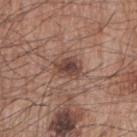No biopsy was performed on this lesion — it was imaged during a full skin examination and was not determined to be concerning.
An algorithmic analysis of the crop reported an area of roughly 6.5 mm², an outline eccentricity of about 0.65 (0 = round, 1 = elongated), and two-axis asymmetry of about 0.25. It also reported a lesion color around L≈44 a*≈19 b*≈24 in CIELAB, roughly 11 lightness units darker than nearby skin, and a lesion-to-skin contrast of about 8.5 (normalized; higher = more distinct). And it measured border irregularity of about 3 on a 0–10 scale, a color-variation rating of about 4.5/10, and a peripheral color-asymmetry measure near 1.5. It also reported a nevus-likeness score of about 70/100 and a detector confidence of about 100 out of 100 that the crop contains a lesion.
A roughly 15 mm field-of-view crop from a total-body skin photograph.
The lesion is on the right upper arm.
Longest diameter approximately 3.5 mm.
A male subject aged around 65.
The tile uses white-light illumination.